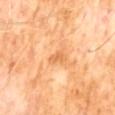{
  "biopsy_status": "not biopsied; imaged during a skin examination",
  "lesion_size": {
    "long_diameter_mm_approx": 2.5
  },
  "patient": {
    "sex": "male",
    "age_approx": 60
  },
  "image": {
    "source": "total-body photography crop",
    "field_of_view_mm": 15
  },
  "automated_metrics": {
    "border_irregularity_0_10": 3.5,
    "peripheral_color_asymmetry": 0.0
  },
  "site": "abdomen"
}